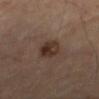The lesion is located on the right thigh. Approximately 3.5 mm at its widest. Automated tile analysis of the lesion measured an outline eccentricity of about 0.7 (0 = round, 1 = elongated) and a shape-asymmetry score of about 0.15 (0 = symmetric). The analysis additionally found a border-irregularity rating of about 1.5/10, internal color variation of about 6 on a 0–10 scale, and a peripheral color-asymmetry measure near 2. The analysis additionally found a classifier nevus-likeness of about 85/100 and lesion-presence confidence of about 100/100. This is a cross-polarized tile. A male subject aged 68 to 72. A 15 mm close-up tile from a total-body photography series done for melanoma screening.